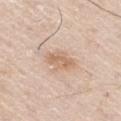Part of a total-body skin-imaging series; this lesion was reviewed on a skin check and was not flagged for biopsy. A 15 mm close-up extracted from a 3D total-body photography capture. The lesion is on the right thigh. A male subject approximately 60 years of age.